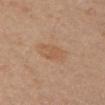Recorded during total-body skin imaging; not selected for excision or biopsy. A roughly 15 mm field-of-view crop from a total-body skin photograph. The patient is a female aged 48–52. Captured under cross-polarized illumination. The total-body-photography lesion software estimated an area of roughly 6.5 mm², an eccentricity of roughly 0.7, and a shape-asymmetry score of about 0.1 (0 = symmetric). The analysis additionally found a mean CIELAB color near L≈53 a*≈18 b*≈31, a lesion–skin lightness drop of about 5, and a normalized border contrast of about 4.5. The analysis additionally found a border-irregularity rating of about 1.5/10 and a peripheral color-asymmetry measure near 1. From the left upper arm. The recorded lesion diameter is about 3.5 mm.